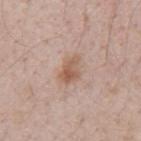{"biopsy_status": "not biopsied; imaged during a skin examination", "patient": {"sex": "male", "age_approx": 40}, "site": "mid back", "image": {"source": "total-body photography crop", "field_of_view_mm": 15}}